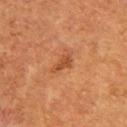<lesion>
  <biopsy_status>not biopsied; imaged during a skin examination</biopsy_status>
  <patient>
    <sex>female</sex>
    <age_approx>40</age_approx>
  </patient>
  <site>upper back</site>
  <lesion_size>
    <long_diameter_mm_approx>3.0</long_diameter_mm_approx>
  </lesion_size>
  <image>
    <source>total-body photography crop</source>
    <field_of_view_mm>15</field_of_view_mm>
  </image>
  <lighting>cross-polarized</lighting>
</lesion>